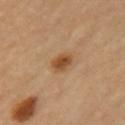{"biopsy_status": "not biopsied; imaged during a skin examination"}